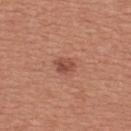The lesion was tiled from a total-body skin photograph and was not biopsied. The subject is a female about 50 years old. A 15 mm crop from a total-body photograph taken for skin-cancer surveillance. On the upper back.